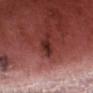Findings:
- biopsy status — imaged on a skin check; not biopsied
- automated lesion analysis — a mean CIELAB color near L≈29 a*≈27 b*≈22 and roughly 11 lightness units darker than nearby skin; a border-irregularity rating of about 3/10, a within-lesion color-variation index near 2/10, and peripheral color asymmetry of about 0.5
- image source — 15 mm crop, total-body photography
- body site — the head or neck
- subject — male, in their mid-70s
- lighting — white-light illumination